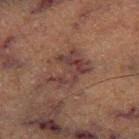Clinical summary: A region of skin cropped from a whole-body photographic capture, roughly 15 mm wide. Located on the right thigh. A male subject in their mid- to late 70s.